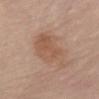biopsy status = total-body-photography surveillance lesion; no biopsy | site = the abdomen | patient = female, aged 68 to 72 | acquisition = ~15 mm crop, total-body skin-cancer survey.